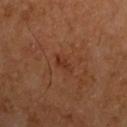Clinical impression:
Captured during whole-body skin photography for melanoma surveillance; the lesion was not biopsied.
Context:
The lesion's longest dimension is about 3 mm. Automated tile analysis of the lesion measured an area of roughly 3 mm², a shape eccentricity near 0.85, and a symmetry-axis asymmetry near 0.4. The analysis additionally found an average lesion color of about L≈32 a*≈24 b*≈30 (CIELAB), roughly 6 lightness units darker than nearby skin, and a normalized lesion–skin contrast near 6. The analysis additionally found an automated nevus-likeness rating near 10 out of 100 and a detector confidence of about 100 out of 100 that the crop contains a lesion. A lesion tile, about 15 mm wide, cut from a 3D total-body photograph. A male patient, about 65 years old. The lesion is located on the left upper arm. Imaged with cross-polarized lighting.